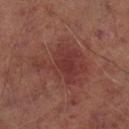Clinical summary: A 15 mm close-up extracted from a 3D total-body photography capture. The lesion is on the left lower leg. The lesion's longest dimension is about 7.5 mm. A male subject aged 63–67. Captured under cross-polarized illumination. Automated image analysis of the tile measured border irregularity of about 5.5 on a 0–10 scale and a color-variation rating of about 4/10.